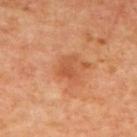About 2.5 mm across. Captured under cross-polarized illumination. From the upper back. A roughly 15 mm field-of-view crop from a total-body skin photograph. A subject aged 63 to 67. An algorithmic analysis of the crop reported a footprint of about 4 mm² and two-axis asymmetry of about 0.45. And it measured a nevus-likeness score of about 0/100 and a detector confidence of about 100 out of 100 that the crop contains a lesion.